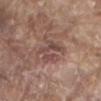Q: Is there a histopathology result?
A: imaged on a skin check; not biopsied
Q: Patient demographics?
A: male, aged 78–82
Q: What is the lesion's diameter?
A: ~4 mm (longest diameter)
Q: How was this image acquired?
A: total-body-photography crop, ~15 mm field of view
Q: Automated lesion metrics?
A: roughly 8 lightness units darker than nearby skin and a normalized border contrast of about 6.5; a border-irregularity index near 7.5/10 and internal color variation of about 5 on a 0–10 scale; a nevus-likeness score of about 0/100 and lesion-presence confidence of about 80/100
Q: What lighting was used for the tile?
A: white-light
Q: Where on the body is the lesion?
A: the mid back